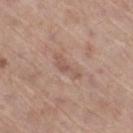This lesion was catalogued during total-body skin photography and was not selected for biopsy. Located on the right thigh. This image is a 15 mm lesion crop taken from a total-body photograph. The tile uses white-light illumination. Longest diameter approximately 4 mm. A male subject aged 68–72.